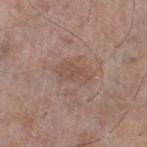Q: Is there a histopathology result?
A: imaged on a skin check; not biopsied
Q: What is the lesion's diameter?
A: ~4 mm (longest diameter)
Q: Lesion location?
A: the left lower leg
Q: How was this image acquired?
A: total-body-photography crop, ~15 mm field of view
Q: What are the patient's age and sex?
A: male, about 60 years old